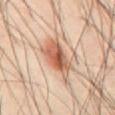The lesion was photographed on a routine skin check and not biopsied; there is no pathology result.
The tile uses cross-polarized illumination.
A region of skin cropped from a whole-body photographic capture, roughly 15 mm wide.
The subject is a male aged 43 to 47.
Located on the abdomen.